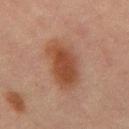• follow-up — total-body-photography surveillance lesion; no biopsy
• patient — male, aged around 65
• anatomic site — the abdomen
• diameter — ≈7 mm
• image-analysis metrics — a mean CIELAB color near L≈37 a*≈19 b*≈26, roughly 9 lightness units darker than nearby skin, and a normalized lesion–skin contrast near 8.5; border irregularity of about 2.5 on a 0–10 scale, internal color variation of about 4 on a 0–10 scale, and radial color variation of about 1; an automated nevus-likeness rating near 100 out of 100 and a detector confidence of about 100 out of 100 that the crop contains a lesion
• lighting — cross-polarized
• image — 15 mm crop, total-body photography